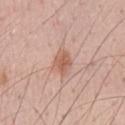<lesion>
<biopsy_status>not biopsied; imaged during a skin examination</biopsy_status>
<patient>
  <sex>male</sex>
  <age_approx>55</age_approx>
</patient>
<lesion_size>
  <long_diameter_mm_approx>2.5</long_diameter_mm_approx>
</lesion_size>
<image>
  <source>total-body photography crop</source>
  <field_of_view_mm>15</field_of_view_mm>
</image>
<site>chest</site>
</lesion>